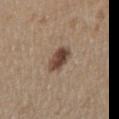The recorded lesion diameter is about 3.5 mm.
Imaged with white-light lighting.
A male patient, aged approximately 55.
From the right upper arm.
A lesion tile, about 15 mm wide, cut from a 3D total-body photograph.